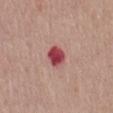notes = no biopsy performed (imaged during a skin exam)
anatomic site = the mid back
patient = female, aged 58–62
imaging modality = 15 mm crop, total-body photography
automated lesion analysis = a lesion area of about 6 mm², a shape eccentricity near 0.6, and two-axis asymmetry of about 0.15; border irregularity of about 1.5 on a 0–10 scale, internal color variation of about 4 on a 0–10 scale, and a peripheral color-asymmetry measure near 1
diameter = ≈3 mm
lighting = white-light illumination